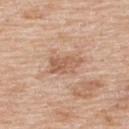- biopsy status — imaged on a skin check; not biopsied
- imaging modality — ~15 mm tile from a whole-body skin photo
- subject — male, in their 60s
- site — the upper back